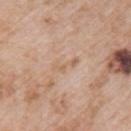The lesion was photographed on a routine skin check and not biopsied; there is no pathology result. Captured under white-light illumination. A close-up tile cropped from a whole-body skin photograph, about 15 mm across. The lesion is located on the arm. About 3.5 mm across. A female subject, roughly 70 years of age. The total-body-photography lesion software estimated a lesion area of about 3 mm², an eccentricity of roughly 0.95, and a shape-asymmetry score of about 0.5 (0 = symmetric). The software also gave an average lesion color of about L≈62 a*≈18 b*≈32 (CIELAB), a lesion–skin lightness drop of about 6, and a normalized lesion–skin contrast near 5. It also reported a classifier nevus-likeness of about 0/100 and lesion-presence confidence of about 90/100.